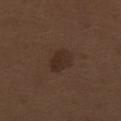Recorded during total-body skin imaging; not selected for excision or biopsy. The lesion is on the right thigh. The patient is a male roughly 70 years of age. A lesion tile, about 15 mm wide, cut from a 3D total-body photograph. Captured under white-light illumination.